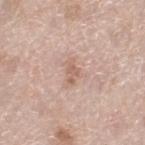Imaged during a routine full-body skin examination; the lesion was not biopsied and no histopathology is available. A female patient, about 60 years old. A 15 mm close-up extracted from a 3D total-body photography capture. The lesion is on the left thigh. An algorithmic analysis of the crop reported a detector confidence of about 100 out of 100 that the crop contains a lesion. This is a white-light tile.